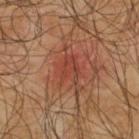Part of a total-body skin-imaging series; this lesion was reviewed on a skin check and was not flagged for biopsy. A lesion tile, about 15 mm wide, cut from a 3D total-body photograph. The total-body-photography lesion software estimated a lesion area of about 11 mm², an eccentricity of roughly 0.8, and a symmetry-axis asymmetry near 0.45. The software also gave roughly 7 lightness units darker than nearby skin and a normalized border contrast of about 6. The analysis additionally found a border-irregularity index near 7/10. The software also gave a nevus-likeness score of about 10/100 and a detector confidence of about 100 out of 100 that the crop contains a lesion. On the upper back. A male patient, approximately 65 years of age. This is a cross-polarized tile. Approximately 6 mm at its widest.